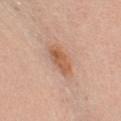Clinical impression:
Recorded during total-body skin imaging; not selected for excision or biopsy.
Clinical summary:
A region of skin cropped from a whole-body photographic capture, roughly 15 mm wide. Measured at roughly 4 mm in maximum diameter. The tile uses white-light illumination. The patient is a female approximately 45 years of age. Located on the chest.